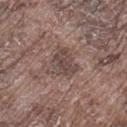follow-up: total-body-photography surveillance lesion; no biopsy | tile lighting: white-light | automated metrics: a mean CIELAB color near L≈45 a*≈16 b*≈19 and a lesion-to-skin contrast of about 6.5 (normalized; higher = more distinct); border irregularity of about 4 on a 0–10 scale, a within-lesion color-variation index near 4/10, and peripheral color asymmetry of about 1 | site: the leg | subject: male, aged around 75 | image: ~15 mm tile from a whole-body skin photo.